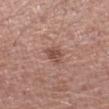workup — imaged on a skin check; not biopsied | image-analysis metrics — a lesion area of about 3.5 mm² and an outline eccentricity of about 0.75 (0 = round, 1 = elongated); a mean CIELAB color near L≈49 a*≈22 b*≈25 and a lesion–skin lightness drop of about 10; a detector confidence of about 100 out of 100 that the crop contains a lesion | patient — male, aged approximately 50 | location — the left upper arm | lesion size — about 2.5 mm | lighting — white-light illumination | image — ~15 mm tile from a whole-body skin photo.